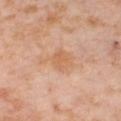The lesion was tiled from a total-body skin photograph and was not biopsied.
A female patient in their mid- to late 50s.
A lesion tile, about 15 mm wide, cut from a 3D total-body photograph.
The total-body-photography lesion software estimated an area of roughly 7 mm² and an eccentricity of roughly 0.65. The software also gave a lesion color around L≈63 a*≈22 b*≈35 in CIELAB, roughly 7 lightness units darker than nearby skin, and a normalized lesion–skin contrast near 5.5. And it measured an automated nevus-likeness rating near 0 out of 100.
From the right thigh.
Approximately 4 mm at its widest.